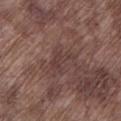Assessment:
This lesion was catalogued during total-body skin photography and was not selected for biopsy.
Image and clinical context:
Imaged with white-light lighting. Located on the left lower leg. Cropped from a whole-body photographic skin survey; the tile spans about 15 mm. A male patient aged 73–77. The lesion-visualizer software estimated a footprint of about 4.5 mm², an eccentricity of roughly 0.75, and a shape-asymmetry score of about 0.55 (0 = symmetric). The software also gave an average lesion color of about L≈38 a*≈18 b*≈19 (CIELAB) and a normalized lesion–skin contrast near 5. The analysis additionally found a classifier nevus-likeness of about 0/100.